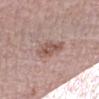No biopsy was performed on this lesion — it was imaged during a full skin examination and was not determined to be concerning.
Imaged with white-light lighting.
On the arm.
The lesion-visualizer software estimated a border-irregularity rating of about 3.5/10, a within-lesion color-variation index near 4/10, and peripheral color asymmetry of about 1.
A 15 mm close-up tile from a total-body photography series done for melanoma screening.
A female subject aged around 65.